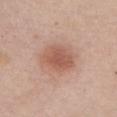Q: Was this lesion biopsied?
A: imaged on a skin check; not biopsied
Q: How was this image acquired?
A: ~15 mm crop, total-body skin-cancer survey
Q: How large is the lesion?
A: about 4 mm
Q: What lighting was used for the tile?
A: white-light
Q: Patient demographics?
A: female, aged 48 to 52
Q: Where on the body is the lesion?
A: the chest
Q: Automated lesion metrics?
A: a border-irregularity rating of about 1.5/10, a color-variation rating of about 3/10, and radial color variation of about 1; a classifier nevus-likeness of about 100/100 and lesion-presence confidence of about 100/100